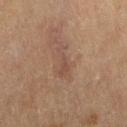Case summary:
* biopsy status: catalogued during a skin exam; not biopsied
* acquisition: 15 mm crop, total-body photography
* body site: the leg
* illumination: cross-polarized
* subject: female, roughly 80 years of age
* lesion size: ~4.5 mm (longest diameter)
* automated metrics: an outline eccentricity of about 0.95 (0 = round, 1 = elongated) and two-axis asymmetry of about 0.55